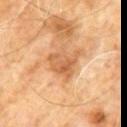<lesion>
  <biopsy_status>not biopsied; imaged during a skin examination</biopsy_status>
  <lesion_size>
    <long_diameter_mm_approx>4.0</long_diameter_mm_approx>
  </lesion_size>
  <image>
    <source>total-body photography crop</source>
    <field_of_view_mm>15</field_of_view_mm>
  </image>
  <lighting>cross-polarized</lighting>
  <site>abdomen</site>
  <automated_metrics>
    <cielab_L>59</cielab_L>
    <cielab_a>24</cielab_a>
    <cielab_b>40</cielab_b>
    <vs_skin_darker_L>10.0</vs_skin_darker_L>
    <border_irregularity_0_10>4.0</border_irregularity_0_10>
    <color_variation_0_10>4.5</color_variation_0_10>
    <peripheral_color_asymmetry>1.5</peripheral_color_asymmetry>
    <nevus_likeness_0_100>0</nevus_likeness_0_100>
    <lesion_detection_confidence_0_100>100</lesion_detection_confidence_0_100>
  </automated_metrics>
  <patient>
    <sex>male</sex>
    <age_approx>60</age_approx>
  </patient>
</lesion>